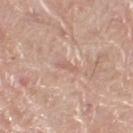Captured during whole-body skin photography for melanoma surveillance; the lesion was not biopsied.
Imaged with white-light lighting.
Longest diameter approximately 2.5 mm.
A 15 mm close-up tile from a total-body photography series done for melanoma screening.
The lesion is on the right thigh.
An algorithmic analysis of the crop reported an outline eccentricity of about 0.95 (0 = round, 1 = elongated) and two-axis asymmetry of about 0.3. It also reported a border-irregularity index near 3.5/10, internal color variation of about 0 on a 0–10 scale, and peripheral color asymmetry of about 0.
A male subject aged 78 to 82.